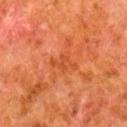Assessment:
No biopsy was performed on this lesion — it was imaged during a full skin examination and was not determined to be concerning.
Clinical summary:
Automated tile analysis of the lesion measured an average lesion color of about L≈40 a*≈29 b*≈36 (CIELAB), about 5 CIELAB-L* units darker than the surrounding skin, and a lesion-to-skin contrast of about 4.5 (normalized; higher = more distinct). Located on the right lower leg. A 15 mm crop from a total-body photograph taken for skin-cancer surveillance. The lesion's longest dimension is about 4 mm. A male patient aged approximately 80.